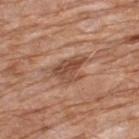Imaged during a routine full-body skin examination; the lesion was not biopsied and no histopathology is available.
Measured at roughly 4.5 mm in maximum diameter.
On the upper back.
A male patient, in their 80s.
A close-up tile cropped from a whole-body skin photograph, about 15 mm across.